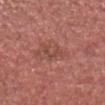  biopsy_status: not biopsied; imaged during a skin examination
  image:
    source: total-body photography crop
    field_of_view_mm: 15
  patient:
    sex: male
    age_approx: 65
  site: head or neck